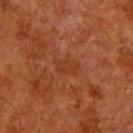The lesion is on the leg.
The recorded lesion diameter is about 3 mm.
This is a cross-polarized tile.
Cropped from a whole-body photographic skin survey; the tile spans about 15 mm.
The patient is a male in their 80s.
Automated tile analysis of the lesion measured a footprint of about 4.5 mm² and a shape eccentricity near 0.8. The analysis additionally found a nevus-likeness score of about 0/100.